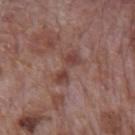Imaged during a routine full-body skin examination; the lesion was not biopsied and no histopathology is available. Located on the mid back. This image is a 15 mm lesion crop taken from a total-body photograph. The recorded lesion diameter is about 4.5 mm. A male subject, in their 70s.